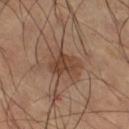The lesion was tiled from a total-body skin photograph and was not biopsied. A region of skin cropped from a whole-body photographic capture, roughly 15 mm wide. Imaged with cross-polarized lighting. The lesion is located on the right thigh. A male patient aged approximately 60.